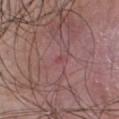Q: Is there a histopathology result?
A: imaged on a skin check; not biopsied
Q: Patient demographics?
A: male, aged around 45
Q: What is the imaging modality?
A: 15 mm crop, total-body photography
Q: How was the tile lit?
A: white-light illumination
Q: Where on the body is the lesion?
A: the upper back